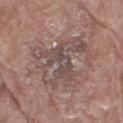Q: Was this lesion biopsied?
A: imaged on a skin check; not biopsied
Q: How was the tile lit?
A: white-light illumination
Q: Lesion location?
A: the leg
Q: Patient demographics?
A: female, aged approximately 70
Q: What is the lesion's diameter?
A: ~6.5 mm (longest diameter)
Q: How was this image acquired?
A: ~15 mm crop, total-body skin-cancer survey